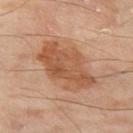The lesion was tiled from a total-body skin photograph and was not biopsied. Imaged with cross-polarized lighting. A roughly 15 mm field-of-view crop from a total-body skin photograph. The subject is a male aged 63–67. The lesion is on the left thigh. Measured at roughly 7.5 mm in maximum diameter. Automated tile analysis of the lesion measured an automated nevus-likeness rating near 20 out of 100 and a lesion-detection confidence of about 100/100.